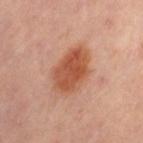Q: Is there a histopathology result?
A: no biopsy performed (imaged during a skin exam)